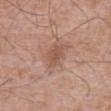Q: Is there a histopathology result?
A: total-body-photography surveillance lesion; no biopsy
Q: What is the anatomic site?
A: the abdomen
Q: Who is the patient?
A: male, aged around 70
Q: Lesion size?
A: about 3 mm
Q: What did automated image analysis measure?
A: roughly 8 lightness units darker than nearby skin and a lesion-to-skin contrast of about 6 (normalized; higher = more distinct); a border-irregularity index near 2.5/10, a within-lesion color-variation index near 1.5/10, and radial color variation of about 0.5
Q: Illumination type?
A: white-light illumination
Q: What is the imaging modality?
A: ~15 mm crop, total-body skin-cancer survey